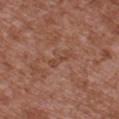workup=total-body-photography surveillance lesion; no biopsy
image-analysis metrics=an area of roughly 3 mm², a shape eccentricity near 0.95, and a symmetry-axis asymmetry near 0.3; an average lesion color of about L≈45 a*≈23 b*≈28 (CIELAB), about 5 CIELAB-L* units darker than the surrounding skin, and a normalized border contrast of about 4.5; a classifier nevus-likeness of about 0/100
patient=male, in their mid- to late 40s
illumination=white-light illumination
imaging modality=total-body-photography crop, ~15 mm field of view
location=the chest